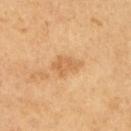Impression: Imaged during a routine full-body skin examination; the lesion was not biopsied and no histopathology is available. Clinical summary: Longest diameter approximately 3.5 mm. Imaged with cross-polarized lighting. A lesion tile, about 15 mm wide, cut from a 3D total-body photograph. Automated image analysis of the tile measured a lesion color around L≈61 a*≈21 b*≈40 in CIELAB, about 9 CIELAB-L* units darker than the surrounding skin, and a lesion-to-skin contrast of about 5.5 (normalized; higher = more distinct). And it measured a border-irregularity rating of about 3/10, a within-lesion color-variation index near 2/10, and radial color variation of about 0.5. The analysis additionally found a detector confidence of about 100 out of 100 that the crop contains a lesion. A male subject aged approximately 65.